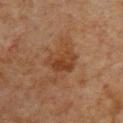| field | value |
|---|---|
| workup | no biopsy performed (imaged during a skin exam) |